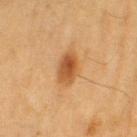biopsy_status: not biopsied; imaged during a skin examination
automated_metrics:
  cielab_L: 46
  cielab_a: 21
  cielab_b: 36
  vs_skin_darker_L: 10.0
site: left thigh
lesion_size:
  long_diameter_mm_approx: 4.0
patient:
  sex: female
  age_approx: 55
image:
  source: total-body photography crop
  field_of_view_mm: 15